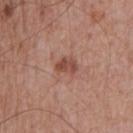{
  "biopsy_status": "not biopsied; imaged during a skin examination"
}